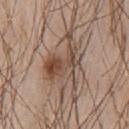A 15 mm close-up tile from a total-body photography series done for melanoma screening. Located on the chest. The recorded lesion diameter is about 9 mm. An algorithmic analysis of the crop reported a border-irregularity rating of about 8/10, a within-lesion color-variation index near 9.5/10, and peripheral color asymmetry of about 3.5. A male subject aged around 40.